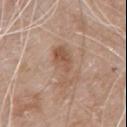Imaged during a routine full-body skin examination; the lesion was not biopsied and no histopathology is available.
The lesion is on the chest.
A lesion tile, about 15 mm wide, cut from a 3D total-body photograph.
The patient is a male aged 78–82.
The tile uses white-light illumination.
The lesion-visualizer software estimated a footprint of about 8 mm², an outline eccentricity of about 0.95 (0 = round, 1 = elongated), and two-axis asymmetry of about 0.5. The software also gave border irregularity of about 6.5 on a 0–10 scale and a color-variation rating of about 3.5/10.
The recorded lesion diameter is about 5.5 mm.